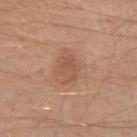Q: Was a biopsy performed?
A: catalogued during a skin exam; not biopsied
Q: Automated lesion metrics?
A: a shape eccentricity near 0.75 and two-axis asymmetry of about 0.2; internal color variation of about 2.5 on a 0–10 scale; a detector confidence of about 100 out of 100 that the crop contains a lesion
Q: Lesion size?
A: ≈3.5 mm
Q: Where on the body is the lesion?
A: the left upper arm
Q: What are the patient's age and sex?
A: male, aged approximately 40
Q: How was this image acquired?
A: 15 mm crop, total-body photography